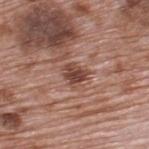Imaged during a routine full-body skin examination; the lesion was not biopsied and no histopathology is available. A roughly 15 mm field-of-view crop from a total-body skin photograph. The recorded lesion diameter is about 3 mm. From the upper back. A male subject aged around 70.